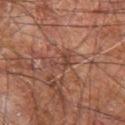On the right leg. A male patient aged approximately 60. Imaged with cross-polarized lighting. Approximately 3 mm at its widest. A close-up tile cropped from a whole-body skin photograph, about 15 mm across. An algorithmic analysis of the crop reported an area of roughly 4 mm² and an outline eccentricity of about 0.75 (0 = round, 1 = elongated). The analysis additionally found a mean CIELAB color near L≈41 a*≈21 b*≈25 and a lesion-to-skin contrast of about 6 (normalized; higher = more distinct). It also reported a nevus-likeness score of about 0/100.